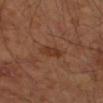Image and clinical context:
The lesion is on the left forearm. A lesion tile, about 15 mm wide, cut from a 3D total-body photograph. A male patient roughly 65 years of age.